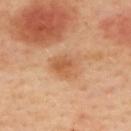workup = total-body-photography surveillance lesion; no biopsy.